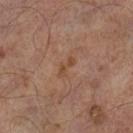Q: Is there a histopathology result?
A: imaged on a skin check; not biopsied
Q: Who is the patient?
A: male, aged approximately 65
Q: Illumination type?
A: cross-polarized
Q: What is the lesion's diameter?
A: about 3 mm
Q: What kind of image is this?
A: ~15 mm tile from a whole-body skin photo
Q: Where on the body is the lesion?
A: the left lower leg